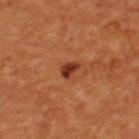Image and clinical context: Approximately 2 mm at its widest. The lesion-visualizer software estimated a lesion color around L≈35 a*≈28 b*≈34 in CIELAB, roughly 12 lightness units darker than nearby skin, and a lesion-to-skin contrast of about 10 (normalized; higher = more distinct). A male subject about 55 years old. A 15 mm close-up tile from a total-body photography series done for melanoma screening. The lesion is on the upper back.